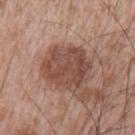Captured during whole-body skin photography for melanoma surveillance; the lesion was not biopsied.
The lesion is on the left upper arm.
A male subject aged 63 to 67.
A 15 mm close-up extracted from a 3D total-body photography capture.